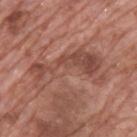The subject is a male aged 68 to 72.
This image is a 15 mm lesion crop taken from a total-body photograph.
This is a white-light tile.
The lesion's longest dimension is about 9 mm.
The lesion is located on the upper back.